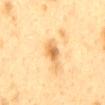notes: total-body-photography surveillance lesion; no biopsy
image source: ~15 mm crop, total-body skin-cancer survey
patient: female, roughly 40 years of age
tile lighting: cross-polarized illumination
lesion diameter: about 3.5 mm
body site: the back